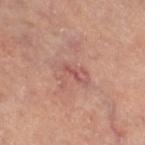The lesion was photographed on a routine skin check and not biopsied; there is no pathology result. Automated image analysis of the tile measured an eccentricity of roughly 0.95 and a shape-asymmetry score of about 0.4 (0 = symmetric). And it measured a mean CIELAB color near L≈53 a*≈28 b*≈24, roughly 8 lightness units darker than nearby skin, and a normalized lesion–skin contrast near 6. The analysis additionally found border irregularity of about 4.5 on a 0–10 scale and peripheral color asymmetry of about 0. Located on the right thigh. A close-up tile cropped from a whole-body skin photograph, about 15 mm across. The tile uses cross-polarized illumination. About 2.5 mm across. A female patient aged around 60.